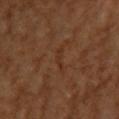This lesion was catalogued during total-body skin photography and was not selected for biopsy. Cropped from a whole-body photographic skin survey; the tile spans about 15 mm. A female subject, aged 63 to 67. On the upper back. This is a cross-polarized tile.